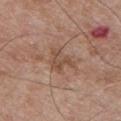No biopsy was performed on this lesion — it was imaged during a full skin examination and was not determined to be concerning. The patient is a male aged around 50. Cropped from a total-body skin-imaging series; the visible field is about 15 mm. Located on the chest.